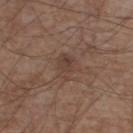Case summary:
– biopsy status: total-body-photography surveillance lesion; no biopsy
– location: the right lower leg
– patient: male, aged 53–57
– image source: 15 mm crop, total-body photography
– image-analysis metrics: a mean CIELAB color near L≈41 a*≈17 b*≈24, about 6 CIELAB-L* units darker than the surrounding skin, and a lesion-to-skin contrast of about 5 (normalized; higher = more distinct); border irregularity of about 3 on a 0–10 scale, internal color variation of about 3 on a 0–10 scale, and radial color variation of about 1.5; an automated nevus-likeness rating near 0 out of 100 and a lesion-detection confidence of about 100/100
– size: ≈3.5 mm
– tile lighting: white-light illumination